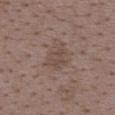The lesion was photographed on a routine skin check and not biopsied; there is no pathology result. On the upper back. A female subject, aged approximately 35. The tile uses white-light illumination. The recorded lesion diameter is about 3.5 mm. Automated image analysis of the tile measured an eccentricity of roughly 0.6 and a symmetry-axis asymmetry near 0.25. The analysis additionally found a mean CIELAB color near L≈46 a*≈15 b*≈23, a lesion–skin lightness drop of about 6, and a normalized lesion–skin contrast near 5.5. The analysis additionally found peripheral color asymmetry of about 0.5. Cropped from a total-body skin-imaging series; the visible field is about 15 mm.